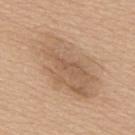biopsy status: no biopsy performed (imaged during a skin exam) | automated metrics: about 8 CIELAB-L* units darker than the surrounding skin and a lesion-to-skin contrast of about 5.5 (normalized; higher = more distinct); a detector confidence of about 100 out of 100 that the crop contains a lesion | tile lighting: white-light | image source: ~15 mm crop, total-body skin-cancer survey | lesion size: ≈9.5 mm | anatomic site: the upper back | subject: male, approximately 60 years of age.